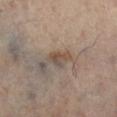Notes:
– workup: catalogued during a skin exam; not biopsied
– site: the left leg
– imaging modality: ~15 mm crop, total-body skin-cancer survey
– subject: male, aged 48 to 52